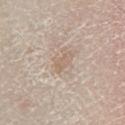A male subject, approximately 60 years of age. Cropped from a total-body skin-imaging series; the visible field is about 15 mm. On the right lower leg.